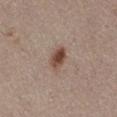| key | value |
|---|---|
| notes | catalogued during a skin exam; not biopsied |
| subject | male, in their mid- to late 70s |
| image-analysis metrics | a shape eccentricity near 0.65 and a shape-asymmetry score of about 0.2 (0 = symmetric) |
| diameter | about 3 mm |
| anatomic site | the right thigh |
| illumination | white-light |
| image source | ~15 mm crop, total-body skin-cancer survey |